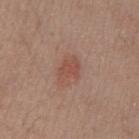notes: imaged on a skin check; not biopsied | automated lesion analysis: a border-irregularity rating of about 3/10, a within-lesion color-variation index near 1.5/10, and radial color variation of about 0.5 | diameter: ≈3 mm | anatomic site: the right upper arm | image: total-body-photography crop, ~15 mm field of view | patient: male, aged 28–32 | lighting: white-light illumination.